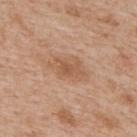biopsy_status: not biopsied; imaged during a skin examination
site: upper back
lighting: white-light
image:
  source: total-body photography crop
  field_of_view_mm: 15
patient:
  sex: male
  age_approx: 65
lesion_size:
  long_diameter_mm_approx: 4.5